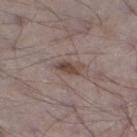Clinical impression:
The lesion was photographed on a routine skin check and not biopsied; there is no pathology result.
Context:
A lesion tile, about 15 mm wide, cut from a 3D total-body photograph. The subject is a male aged around 70. The tile uses white-light illumination. Located on the leg.